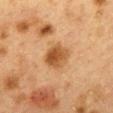Captured during whole-body skin photography for melanoma surveillance; the lesion was not biopsied. This image is a 15 mm lesion crop taken from a total-body photograph. On the back. A female subject, approximately 40 years of age.